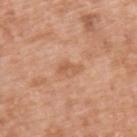Findings:
- notes · imaged on a skin check; not biopsied
- body site · the upper back
- automated metrics · a mean CIELAB color near L≈58 a*≈24 b*≈35, roughly 8 lightness units darker than nearby skin, and a normalized lesion–skin contrast near 5.5; a border-irregularity rating of about 6/10, a within-lesion color-variation index near 0/10, and a peripheral color-asymmetry measure near 0; a classifier nevus-likeness of about 0/100 and a detector confidence of about 100 out of 100 that the crop contains a lesion
- patient · male, aged around 50
- lighting · white-light
- lesion diameter · ≈3 mm
- imaging modality · 15 mm crop, total-body photography